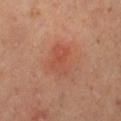Q: Was this lesion biopsied?
A: imaged on a skin check; not biopsied
Q: What lighting was used for the tile?
A: cross-polarized illumination
Q: What kind of image is this?
A: 15 mm crop, total-body photography
Q: Patient demographics?
A: female, aged 38 to 42
Q: Lesion size?
A: about 4 mm
Q: What is the anatomic site?
A: the left thigh
Q: What did automated image analysis measure?
A: a border-irregularity index near 4.5/10 and a color-variation rating of about 4.5/10; a classifier nevus-likeness of about 40/100